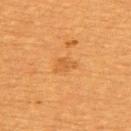Part of a total-body skin-imaging series; this lesion was reviewed on a skin check and was not flagged for biopsy. The lesion is on the upper back. A region of skin cropped from a whole-body photographic capture, roughly 15 mm wide. A female subject aged 53–57.